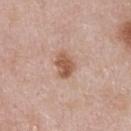<case>
  <biopsy_status>not biopsied; imaged during a skin examination</biopsy_status>
  <site>chest</site>
  <automated_metrics>
    <area_mm2_approx>6.5</area_mm2_approx>
    <eccentricity>0.7</eccentricity>
    <shape_asymmetry>0.25</shape_asymmetry>
    <nevus_likeness_0_100>90</nevus_likeness_0_100>
    <lesion_detection_confidence_0_100>100</lesion_detection_confidence_0_100>
  </automated_metrics>
  <image>
    <source>total-body photography crop</source>
    <field_of_view_mm>15</field_of_view_mm>
  </image>
  <patient>
    <sex>male</sex>
    <age_approx>55</age_approx>
  </patient>
</case>